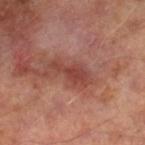Notes:
* image source · ~15 mm crop, total-body skin-cancer survey
* subject · male, aged 63–67
* anatomic site · the leg